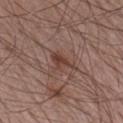{
  "biopsy_status": "not biopsied; imaged during a skin examination",
  "lesion_size": {
    "long_diameter_mm_approx": 3.0
  },
  "site": "left forearm",
  "image": {
    "source": "total-body photography crop",
    "field_of_view_mm": 15
  },
  "patient": {
    "sex": "male",
    "age_approx": 65
  },
  "lighting": "white-light",
  "automated_metrics": {
    "nevus_likeness_0_100": 30,
    "lesion_detection_confidence_0_100": 100
  }
}